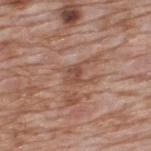– notes — total-body-photography surveillance lesion; no biopsy
– lesion size — about 3.5 mm
– site — the upper back
– lighting — white-light
– image source — 15 mm crop, total-body photography
– automated metrics — a footprint of about 5 mm², an outline eccentricity of about 0.7 (0 = round, 1 = elongated), and a shape-asymmetry score of about 0.4 (0 = symmetric)
– patient — male, aged 58–62